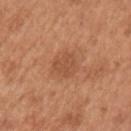  biopsy_status: not biopsied; imaged during a skin examination
  image:
    source: total-body photography crop
    field_of_view_mm: 15
  site: arm
  patient:
    sex: male
    age_approx: 65
  lesion_size:
    long_diameter_mm_approx: 3.5
  lighting: white-light